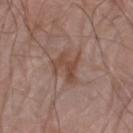Recorded during total-body skin imaging; not selected for excision or biopsy.
Located on the right arm.
The total-body-photography lesion software estimated a lesion area of about 8.5 mm², an outline eccentricity of about 0.7 (0 = round, 1 = elongated), and a shape-asymmetry score of about 0.5 (0 = symmetric). The analysis additionally found a mean CIELAB color near L≈47 a*≈19 b*≈26, a lesion–skin lightness drop of about 8, and a lesion-to-skin contrast of about 7 (normalized; higher = more distinct). And it measured an automated nevus-likeness rating near 0 out of 100 and lesion-presence confidence of about 100/100.
A lesion tile, about 15 mm wide, cut from a 3D total-body photograph.
A male patient about 80 years old.